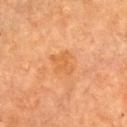Automated image analysis of the tile measured a footprint of about 6 mm², an eccentricity of roughly 0.5, and a symmetry-axis asymmetry near 0.25. Approximately 3 mm at its widest. The tile uses cross-polarized illumination. On the chest. A 15 mm close-up extracted from a 3D total-body photography capture. A female patient about 60 years old.